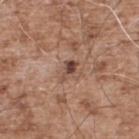<case>
<biopsy_status>not biopsied; imaged during a skin examination</biopsy_status>
<site>upper back</site>
<image>
  <source>total-body photography crop</source>
  <field_of_view_mm>15</field_of_view_mm>
</image>
<patient>
  <sex>male</sex>
  <age_approx>55</age_approx>
</patient>
<lighting>white-light</lighting>
<lesion_size>
  <long_diameter_mm_approx>2.5</long_diameter_mm_approx>
</lesion_size>
</case>